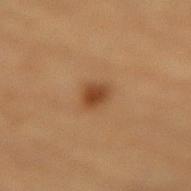Clinical impression: No biopsy was performed on this lesion — it was imaged during a full skin examination and was not determined to be concerning. Context: The total-body-photography lesion software estimated a lesion area of about 4 mm², an outline eccentricity of about 0.6 (0 = round, 1 = elongated), and a shape-asymmetry score of about 0.25 (0 = symmetric). And it measured a border-irregularity index near 2/10 and peripheral color asymmetry of about 1. It also reported lesion-presence confidence of about 100/100. The lesion is on the lower back. A region of skin cropped from a whole-body photographic capture, roughly 15 mm wide. Longest diameter approximately 2.5 mm. A male subject, aged 83 or older.